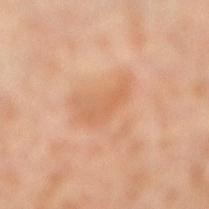Assessment: The lesion was tiled from a total-body skin photograph and was not biopsied. Clinical summary: Approximately 4 mm at its widest. Cropped from a total-body skin-imaging series; the visible field is about 15 mm. Imaged with cross-polarized lighting. A male patient about 55 years old. From the right lower leg.